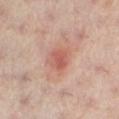{
  "biopsy_status": "not biopsied; imaged during a skin examination",
  "site": "right leg",
  "image": {
    "source": "total-body photography crop",
    "field_of_view_mm": 15
  },
  "lesion_size": {
    "long_diameter_mm_approx": 2.5
  },
  "patient": {
    "sex": "female",
    "age_approx": 40
  },
  "automated_metrics": {
    "cielab_L": 57,
    "cielab_a": 28,
    "cielab_b": 28,
    "vs_skin_darker_L": 9.0,
    "vs_skin_contrast_norm": 6.0
  }
}